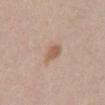| key | value |
|---|---|
| follow-up | catalogued during a skin exam; not biopsied |
| TBP lesion metrics | an area of roughly 4.5 mm², an eccentricity of roughly 0.75, and two-axis asymmetry of about 0.25; an average lesion color of about L≈58 a*≈18 b*≈30 (CIELAB), a lesion–skin lightness drop of about 9, and a normalized lesion–skin contrast near 7 |
| image | 15 mm crop, total-body photography |
| tile lighting | white-light |
| patient | male, approximately 60 years of age |
| site | the front of the torso |